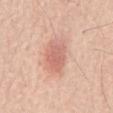The recorded lesion diameter is about 3.5 mm. From the back. The subject is a male aged approximately 70. A 15 mm close-up extracted from a 3D total-body photography capture. This is a white-light tile.